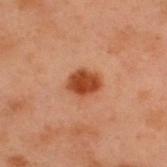Recorded during total-body skin imaging; not selected for excision or biopsy.
A region of skin cropped from a whole-body photographic capture, roughly 15 mm wide.
Located on the back.
Approximately 3.5 mm at its widest.
Automated image analysis of the tile measured a lesion area of about 7.5 mm², an eccentricity of roughly 0.6, and a symmetry-axis asymmetry near 0.15. The software also gave border irregularity of about 1 on a 0–10 scale, a within-lesion color-variation index near 2.5/10, and a peripheral color-asymmetry measure near 1.
The patient is a male in their mid-50s.
Imaged with cross-polarized lighting.